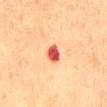workup: no biopsy performed (imaged during a skin exam)
acquisition: ~15 mm crop, total-body skin-cancer survey
patient: female, aged 53–57
anatomic site: the mid back